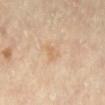Q: Is there a histopathology result?
A: total-body-photography surveillance lesion; no biopsy
Q: What are the patient's age and sex?
A: female, aged 63 to 67
Q: Lesion size?
A: ~2.5 mm (longest diameter)
Q: What kind of image is this?
A: ~15 mm tile from a whole-body skin photo
Q: What did automated image analysis measure?
A: a shape eccentricity near 0.8 and a symmetry-axis asymmetry near 0.4; about 6 CIELAB-L* units darker than the surrounding skin and a lesion-to-skin contrast of about 5 (normalized; higher = more distinct)
Q: Lesion location?
A: the left lower leg
Q: Illumination type?
A: cross-polarized illumination